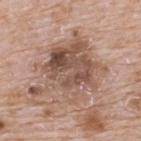The lesion was photographed on a routine skin check and not biopsied; there is no pathology result. A male subject, roughly 65 years of age. Located on the upper back. The total-body-photography lesion software estimated a border-irregularity index near 6.5/10, a within-lesion color-variation index near 7.5/10, and a peripheral color-asymmetry measure near 2.5. Longest diameter approximately 8.5 mm. A 15 mm close-up extracted from a 3D total-body photography capture. The tile uses white-light illumination.